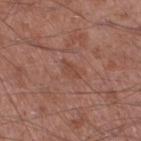Assessment:
Recorded during total-body skin imaging; not selected for excision or biopsy.
Background:
Captured under white-light illumination. The lesion is located on the right thigh. A male patient, in their mid- to late 50s. Measured at roughly 2.5 mm in maximum diameter. A close-up tile cropped from a whole-body skin photograph, about 15 mm across.